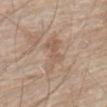biopsy status — total-body-photography surveillance lesion; no biopsy
site — the abdomen
acquisition — ~15 mm crop, total-body skin-cancer survey
diameter — about 5.5 mm
automated lesion analysis — an area of roughly 10 mm² and a shape eccentricity near 0.85; a lesion color around L≈58 a*≈16 b*≈27 in CIELAB, about 6 CIELAB-L* units darker than the surrounding skin, and a normalized border contrast of about 4.5; a nevus-likeness score of about 0/100 and lesion-presence confidence of about 75/100
tile lighting — white-light illumination
patient — male, approximately 80 years of age